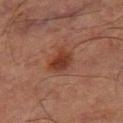biopsy_status: not biopsied; imaged during a skin examination
image:
  source: total-body photography crop
  field_of_view_mm: 15
site: left thigh
patient:
  sex: male
  age_approx: 65